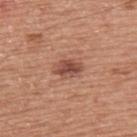Impression: Recorded during total-body skin imaging; not selected for excision or biopsy. Image and clinical context: A 15 mm close-up extracted from a 3D total-body photography capture. Imaged with white-light lighting. A male patient approximately 75 years of age. The lesion is located on the back.